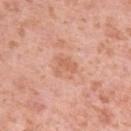biopsy status — no biopsy performed (imaged during a skin exam)
imaging modality — ~15 mm tile from a whole-body skin photo
illumination — white-light
TBP lesion metrics — a footprint of about 4 mm² and two-axis asymmetry of about 0.5; a lesion color around L≈62 a*≈25 b*≈34 in CIELAB, roughly 7 lightness units darker than nearby skin, and a normalized border contrast of about 5.5
subject — female, aged around 40
body site — the left upper arm